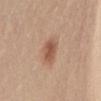follow-up: imaged on a skin check; not biopsied
subject: female, about 30 years old
size: about 3.5 mm
tile lighting: white-light
image source: 15 mm crop, total-body photography
body site: the chest
automated metrics: a footprint of about 6 mm², a shape eccentricity near 0.85, and a shape-asymmetry score of about 0.2 (0 = symmetric); an automated nevus-likeness rating near 100 out of 100 and a lesion-detection confidence of about 100/100